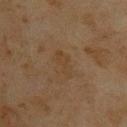Assessment: This lesion was catalogued during total-body skin photography and was not selected for biopsy. Context: Automated tile analysis of the lesion measured a lesion area of about 4.5 mm² and a shape eccentricity near 0.85. The software also gave border irregularity of about 4.5 on a 0–10 scale. A close-up tile cropped from a whole-body skin photograph, about 15 mm across. A male patient aged 43 to 47. From the upper back. Measured at roughly 3.5 mm in maximum diameter. Captured under cross-polarized illumination.